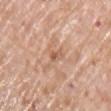<case>
<biopsy_status>not biopsied; imaged during a skin examination</biopsy_status>
<patient>
  <sex>male</sex>
  <age_approx>75</age_approx>
</patient>
<automated_metrics>
  <area_mm2_approx>2.0</area_mm2_approx>
  <eccentricity>0.95</eccentricity>
  <lesion_detection_confidence_0_100>95</lesion_detection_confidence_0_100>
</automated_metrics>
<image>
  <source>total-body photography crop</source>
  <field_of_view_mm>15</field_of_view_mm>
</image>
<lesion_size>
  <long_diameter_mm_approx>2.5</long_diameter_mm_approx>
</lesion_size>
<site>left upper arm</site>
</case>